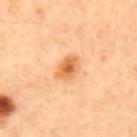{"site": "mid back", "lesion_size": {"long_diameter_mm_approx": 3.5}, "image": {"source": "total-body photography crop", "field_of_view_mm": 15}, "patient": {"sex": "male", "age_approx": 65}, "lighting": "cross-polarized"}